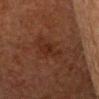Captured during whole-body skin photography for melanoma surveillance; the lesion was not biopsied. A 15 mm crop from a total-body photograph taken for skin-cancer surveillance. This is a cross-polarized tile. From the head or neck. A male subject in their mid- to late 60s. Automated tile analysis of the lesion measured a lesion area of about 4.5 mm² and a shape eccentricity near 0.75. The software also gave border irregularity of about 4.5 on a 0–10 scale, a within-lesion color-variation index near 1.5/10, and radial color variation of about 0.5. It also reported an automated nevus-likeness rating near 5 out of 100 and a lesion-detection confidence of about 100/100.